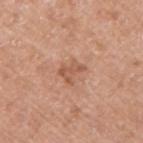Clinical impression: The lesion was tiled from a total-body skin photograph and was not biopsied. Clinical summary: Imaged with white-light lighting. The patient is a male aged 53–57. Located on the right upper arm. A 15 mm crop from a total-body photograph taken for skin-cancer surveillance.